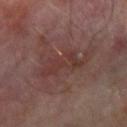Assessment:
Part of a total-body skin-imaging series; this lesion was reviewed on a skin check and was not flagged for biopsy.
Background:
A male subject aged 68 to 72. Automated image analysis of the tile measured about 6 CIELAB-L* units darker than the surrounding skin and a lesion-to-skin contrast of about 5.5 (normalized; higher = more distinct). The software also gave a nevus-likeness score of about 0/100 and a detector confidence of about 100 out of 100 that the crop contains a lesion. A lesion tile, about 15 mm wide, cut from a 3D total-body photograph. On the arm. The lesion's longest dimension is about 5.5 mm. This is a cross-polarized tile.